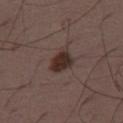<record>
<biopsy_status>not biopsied; imaged during a skin examination</biopsy_status>
<site>right thigh</site>
<lesion_size>
  <long_diameter_mm_approx>3.5</long_diameter_mm_approx>
</lesion_size>
<lighting>white-light</lighting>
<image>
  <source>total-body photography crop</source>
  <field_of_view_mm>15</field_of_view_mm>
</image>
<automated_metrics>
  <area_mm2_approx>6.5</area_mm2_approx>
  <shape_asymmetry>0.25</shape_asymmetry>
  <border_irregularity_0_10>2.0</border_irregularity_0_10>
  <color_variation_0_10>4.0</color_variation_0_10>
  <peripheral_color_asymmetry>1.5</peripheral_color_asymmetry>
  <nevus_likeness_0_100>95</nevus_likeness_0_100>
  <lesion_detection_confidence_0_100>100</lesion_detection_confidence_0_100>
</automated_metrics>
<patient>
  <sex>male</sex>
  <age_approx>50</age_approx>
</patient>
</record>